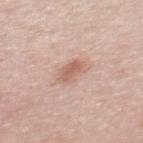follow-up: imaged on a skin check; not biopsied
location: the upper back
image: ~15 mm crop, total-body skin-cancer survey
patient: female, approximately 35 years of age